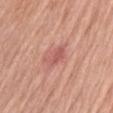notes=imaged on a skin check; not biopsied | TBP lesion metrics=an area of roughly 4.5 mm², an eccentricity of roughly 0.75, and two-axis asymmetry of about 0.15; a normalized border contrast of about 6; a border-irregularity index near 2/10 and internal color variation of about 2.5 on a 0–10 scale | subject=male, about 60 years old | illumination=white-light | image source=total-body-photography crop, ~15 mm field of view | location=the left upper arm | size=~2.5 mm (longest diameter).